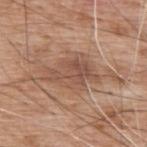{
  "biopsy_status": "not biopsied; imaged during a skin examination",
  "patient": {
    "sex": "male",
    "age_approx": 60
  },
  "site": "upper back",
  "image": {
    "source": "total-body photography crop",
    "field_of_view_mm": 15
  },
  "automated_metrics": {
    "eccentricity": 0.75,
    "shape_asymmetry": 0.3,
    "cielab_L": 51,
    "cielab_a": 20,
    "cielab_b": 28,
    "vs_skin_contrast_norm": 6.5,
    "border_irregularity_0_10": 6.0,
    "peripheral_color_asymmetry": 1.0,
    "nevus_likeness_0_100": 0,
    "lesion_detection_confidence_0_100": 95
  },
  "lighting": "white-light",
  "lesion_size": {
    "long_diameter_mm_approx": 5.5
  }
}